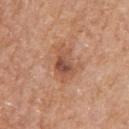Acquisition and patient details:
This image is a 15 mm lesion crop taken from a total-body photograph. About 4 mm across. The tile uses white-light illumination. A male subject, roughly 65 years of age. The lesion is located on the arm. Automated tile analysis of the lesion measured an average lesion color of about L≈52 a*≈23 b*≈32 (CIELAB), about 10 CIELAB-L* units darker than the surrounding skin, and a normalized border contrast of about 7. The analysis additionally found a border-irregularity index near 3/10, internal color variation of about 6 on a 0–10 scale, and radial color variation of about 1.5.